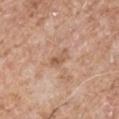Recorded during total-body skin imaging; not selected for excision or biopsy. Measured at roughly 3 mm in maximum diameter. This is a white-light tile. From the right upper arm. Cropped from a whole-body photographic skin survey; the tile spans about 15 mm. The patient is a male roughly 55 years of age. The total-body-photography lesion software estimated a footprint of about 3.5 mm², an outline eccentricity of about 0.85 (0 = round, 1 = elongated), and a symmetry-axis asymmetry near 0.35.